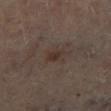{"biopsy_status": "not biopsied; imaged during a skin examination", "image": {"source": "total-body photography crop", "field_of_view_mm": 15}, "patient": {"sex": "female", "age_approx": 60}, "site": "right lower leg"}